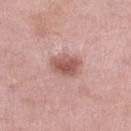{
  "biopsy_status": "not biopsied; imaged during a skin examination",
  "lesion_size": {
    "long_diameter_mm_approx": 3.5
  },
  "patient": {
    "sex": "female",
    "age_approx": 70
  },
  "site": "left lower leg",
  "image": {
    "source": "total-body photography crop",
    "field_of_view_mm": 15
  }
}